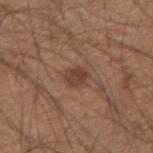notes = no biopsy performed (imaged during a skin exam)
acquisition = ~15 mm crop, total-body skin-cancer survey
location = the right upper arm
subject = male, roughly 35 years of age